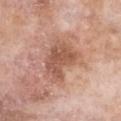No biopsy was performed on this lesion — it was imaged during a full skin examination and was not determined to be concerning. Imaged with white-light lighting. A roughly 15 mm field-of-view crop from a total-body skin photograph. Longest diameter approximately 5 mm. From the chest. The subject is a female in their mid-70s.